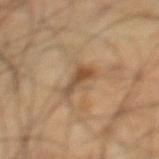This lesion was catalogued during total-body skin photography and was not selected for biopsy. Imaged with cross-polarized lighting. From the left lower leg. Cropped from a total-body skin-imaging series; the visible field is about 15 mm. Automated image analysis of the tile measured a lesion area of about 4 mm², an outline eccentricity of about 0.85 (0 = round, 1 = elongated), and a symmetry-axis asymmetry near 0.5. It also reported a classifier nevus-likeness of about 15/100. The subject is a male aged around 50.